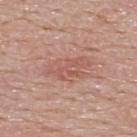The lesion was photographed on a routine skin check and not biopsied; there is no pathology result. Located on the upper back. A lesion tile, about 15 mm wide, cut from a 3D total-body photograph. Measured at roughly 5.5 mm in maximum diameter. A male patient roughly 55 years of age. Imaged with white-light lighting. An algorithmic analysis of the crop reported a lesion area of about 9.5 mm², an outline eccentricity of about 0.9 (0 = round, 1 = elongated), and two-axis asymmetry of about 0.3. The software also gave an average lesion color of about L≈56 a*≈24 b*≈26 (CIELAB), roughly 7 lightness units darker than nearby skin, and a normalized border contrast of about 5. And it measured a border-irregularity index near 4.5/10, a color-variation rating of about 3/10, and a peripheral color-asymmetry measure near 1. And it measured a lesion-detection confidence of about 100/100.